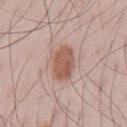Assessment:
This lesion was catalogued during total-body skin photography and was not selected for biopsy.
Acquisition and patient details:
The patient is a male about 45 years old. The tile uses white-light illumination. A close-up tile cropped from a whole-body skin photograph, about 15 mm across. The lesion is on the abdomen. The total-body-photography lesion software estimated a lesion color around L≈57 a*≈20 b*≈27 in CIELAB, about 11 CIELAB-L* units darker than the surrounding skin, and a normalized lesion–skin contrast near 8. It also reported a border-irregularity rating of about 2/10, internal color variation of about 3 on a 0–10 scale, and radial color variation of about 1. And it measured an automated nevus-likeness rating near 95 out of 100 and a lesion-detection confidence of about 100/100.